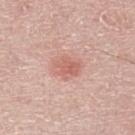The lesion was tiled from a total-body skin photograph and was not biopsied. The recorded lesion diameter is about 3 mm. From the left lower leg. A male patient, aged around 70. A roughly 15 mm field-of-view crop from a total-body skin photograph.